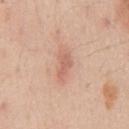Part of a total-body skin-imaging series; this lesion was reviewed on a skin check and was not flagged for biopsy.
A 15 mm close-up tile from a total-body photography series done for melanoma screening.
A male subject in their mid-40s.
Measured at roughly 4 mm in maximum diameter.
Located on the chest.
Captured under white-light illumination.